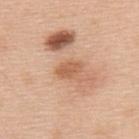Q: Is there a histopathology result?
A: imaged on a skin check; not biopsied
Q: What is the imaging modality?
A: ~15 mm crop, total-body skin-cancer survey
Q: How large is the lesion?
A: about 3 mm
Q: What did automated image analysis measure?
A: a lesion color around L≈59 a*≈23 b*≈35 in CIELAB, roughly 9 lightness units darker than nearby skin, and a lesion-to-skin contrast of about 6.5 (normalized; higher = more distinct); a border-irregularity rating of about 2.5/10, a within-lesion color-variation index near 2/10, and radial color variation of about 1; a nevus-likeness score of about 0/100 and lesion-presence confidence of about 100/100
Q: What are the patient's age and sex?
A: male, aged 48 to 52
Q: What lighting was used for the tile?
A: white-light illumination
Q: Lesion location?
A: the upper back